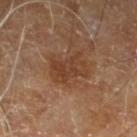<tbp_lesion>
<site>leg</site>
<lesion_size>
  <long_diameter_mm_approx>4.5</long_diameter_mm_approx>
</lesion_size>
<image>
  <source>total-body photography crop</source>
  <field_of_view_mm>15</field_of_view_mm>
</image>
<patient>
  <sex>male</sex>
  <age_approx>70</age_approx>
</patient>
<lighting>cross-polarized</lighting>
</tbp_lesion>